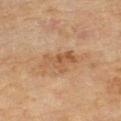workup: total-body-photography surveillance lesion; no biopsy
illumination: cross-polarized illumination
body site: the left thigh
patient: female, aged 58–62
size: about 4 mm
imaging modality: total-body-photography crop, ~15 mm field of view
TBP lesion metrics: a lesion area of about 7 mm²; a mean CIELAB color near L≈50 a*≈19 b*≈34 and a normalized lesion–skin contrast near 6; an automated nevus-likeness rating near 0 out of 100